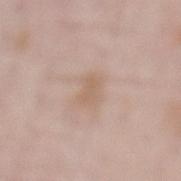Impression:
The lesion was tiled from a total-body skin photograph and was not biopsied.
Context:
On the lower back. A region of skin cropped from a whole-body photographic capture, roughly 15 mm wide. The recorded lesion diameter is about 3 mm. The patient is a male aged around 55.